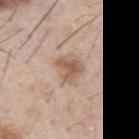Impression:
Recorded during total-body skin imaging; not selected for excision or biopsy.
Image and clinical context:
Longest diameter approximately 4 mm. The lesion-visualizer software estimated a within-lesion color-variation index near 4.5/10 and radial color variation of about 1.5. It also reported a classifier nevus-likeness of about 45/100 and lesion-presence confidence of about 100/100. The subject is a male about 55 years old. A 15 mm crop from a total-body photograph taken for skin-cancer surveillance. The tile uses white-light illumination.